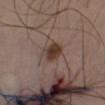The lesion was photographed on a routine skin check and not biopsied; there is no pathology result. A region of skin cropped from a whole-body photographic capture, roughly 15 mm wide. Approximately 3 mm at its widest. A male subject about 40 years old. The lesion is on the chest.